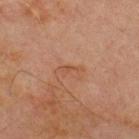| feature | finding |
|---|---|
| biopsy status | total-body-photography surveillance lesion; no biopsy |
| anatomic site | the chest |
| acquisition | ~15 mm crop, total-body skin-cancer survey |
| tile lighting | cross-polarized |
| diameter | ≈2.5 mm |
| TBP lesion metrics | a mean CIELAB color near L≈41 a*≈19 b*≈28 and a normalized border contrast of about 5; a nevus-likeness score of about 0/100 |
| patient | male, roughly 70 years of age |